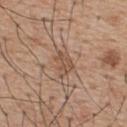Notes:
- biopsy status — catalogued during a skin exam; not biopsied
- subject — male, aged 53–57
- lesion size — ~3 mm (longest diameter)
- illumination — white-light illumination
- imaging modality — ~15 mm crop, total-body skin-cancer survey
- site — the back
- automated metrics — a border-irregularity index near 5/10 and a peripheral color-asymmetry measure near 1; lesion-presence confidence of about 95/100